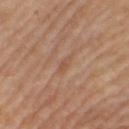No biopsy was performed on this lesion — it was imaged during a full skin examination and was not determined to be concerning.
The lesion is on the right thigh.
About 2.5 mm across.
A female patient, aged 68 to 72.
The tile uses cross-polarized illumination.
A region of skin cropped from a whole-body photographic capture, roughly 15 mm wide.